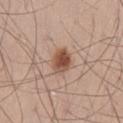Q: Is there a histopathology result?
A: catalogued during a skin exam; not biopsied
Q: Where on the body is the lesion?
A: the leg
Q: Patient demographics?
A: male, aged around 50
Q: How was this image acquired?
A: 15 mm crop, total-body photography